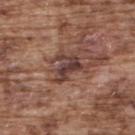Case summary:
* follow-up: total-body-photography surveillance lesion; no biopsy
* anatomic site: the upper back
* image: ~15 mm crop, total-body skin-cancer survey
* patient: male, in their mid- to late 70s